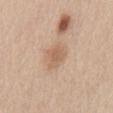Captured during whole-body skin photography for melanoma surveillance; the lesion was not biopsied. Measured at roughly 3.5 mm in maximum diameter. This is a white-light tile. From the abdomen. The patient is a male approximately 60 years of age. Cropped from a whole-body photographic skin survey; the tile spans about 15 mm.